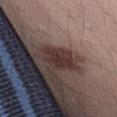No biopsy was performed on this lesion — it was imaged during a full skin examination and was not determined to be concerning. Imaged with white-light lighting. Cropped from a total-body skin-imaging series; the visible field is about 15 mm. The subject is a male aged 28–32.